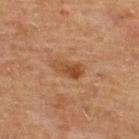| key | value |
|---|---|
| automated lesion analysis | an outline eccentricity of about 0.85 (0 = round, 1 = elongated); an average lesion color of about L≈42 a*≈21 b*≈33 (CIELAB), about 9 CIELAB-L* units darker than the surrounding skin, and a normalized border contrast of about 7.5; a lesion-detection confidence of about 100/100 |
| anatomic site | the upper back |
| patient | male, approximately 65 years of age |
| illumination | cross-polarized |
| imaging modality | ~15 mm tile from a whole-body skin photo |
| lesion diameter | about 3.5 mm |